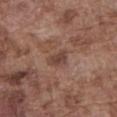Case summary:
• follow-up · total-body-photography surveillance lesion; no biopsy
• subject · male, in their mid- to late 70s
• location · the abdomen
• image · ~15 mm crop, total-body skin-cancer survey
• tile lighting · white-light illumination
• size · ~2.5 mm (longest diameter)